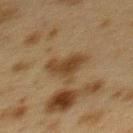Clinical impression:
Captured during whole-body skin photography for melanoma surveillance; the lesion was not biopsied.
Context:
This image is a 15 mm lesion crop taken from a total-body photograph. The subject is a female roughly 40 years of age. Measured at roughly 4.5 mm in maximum diameter. The lesion is located on the back.